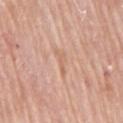Clinical impression:
No biopsy was performed on this lesion — it was imaged during a full skin examination and was not determined to be concerning.
Acquisition and patient details:
The tile uses white-light illumination. A roughly 15 mm field-of-view crop from a total-body skin photograph. An algorithmic analysis of the crop reported an average lesion color of about L≈64 a*≈21 b*≈32 (CIELAB) and roughly 6 lightness units darker than nearby skin. The analysis additionally found a classifier nevus-likeness of about 0/100. The lesion is located on the right upper arm. A female subject aged approximately 65. The lesion's longest dimension is about 3 mm.